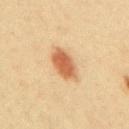This lesion was catalogued during total-body skin photography and was not selected for biopsy. A region of skin cropped from a whole-body photographic capture, roughly 15 mm wide. The lesion is located on the upper back. A male patient, roughly 40 years of age.